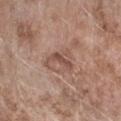Assessment: Recorded during total-body skin imaging; not selected for excision or biopsy.